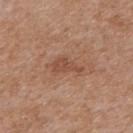Background: Approximately 4 mm at its widest. A close-up tile cropped from a whole-body skin photograph, about 15 mm across. The tile uses white-light illumination. On the upper back. A female subject approximately 75 years of age.